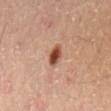{"biopsy_status": "not biopsied; imaged during a skin examination", "patient": {"sex": "male", "age_approx": 55}, "image": {"source": "total-body photography crop", "field_of_view_mm": 15}, "site": "back"}